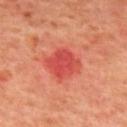Located on the mid back. A roughly 15 mm field-of-view crop from a total-body skin photograph. The total-body-photography lesion software estimated a footprint of about 10 mm², an eccentricity of roughly 0.4, and two-axis asymmetry of about 0.15. And it measured border irregularity of about 1.5 on a 0–10 scale, internal color variation of about 3.5 on a 0–10 scale, and peripheral color asymmetry of about 1. Captured under cross-polarized illumination. A male subject aged approximately 65. About 3.5 mm across.